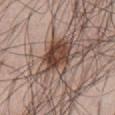follow-up = total-body-photography surveillance lesion; no biopsy
lesion size = ~6 mm (longest diameter)
anatomic site = the abdomen
imaging modality = 15 mm crop, total-body photography
automated metrics = a lesion color around L≈45 a*≈17 b*≈24 in CIELAB, roughly 14 lightness units darker than nearby skin, and a normalized lesion–skin contrast near 10; a border-irregularity index near 4/10 and a peripheral color-asymmetry measure near 3; a nevus-likeness score of about 80/100 and lesion-presence confidence of about 100/100
subject = male, aged approximately 70